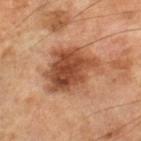Recorded during total-body skin imaging; not selected for excision or biopsy. Cropped from a whole-body photographic skin survey; the tile spans about 15 mm. Automated image analysis of the tile measured an area of roughly 24 mm² and a shape-asymmetry score of about 0.3 (0 = symmetric). The software also gave an automated nevus-likeness rating near 70 out of 100 and lesion-presence confidence of about 100/100. A male patient approximately 65 years of age. On the leg.